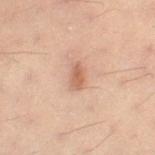Imaged during a routine full-body skin examination; the lesion was not biopsied and no histopathology is available. The subject is a female aged 28 to 32. A close-up tile cropped from a whole-body skin photograph, about 15 mm across. Measured at roughly 2.5 mm in maximum diameter. The tile uses cross-polarized illumination. An algorithmic analysis of the crop reported a lesion area of about 3 mm², a shape eccentricity near 0.85, and two-axis asymmetry of about 0.2. The analysis additionally found a lesion color around L≈48 a*≈19 b*≈26 in CIELAB, about 9 CIELAB-L* units darker than the surrounding skin, and a lesion-to-skin contrast of about 7 (normalized; higher = more distinct). It also reported a nevus-likeness score of about 65/100 and lesion-presence confidence of about 100/100. Located on the leg.